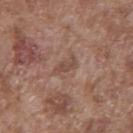Impression: No biopsy was performed on this lesion — it was imaged during a full skin examination and was not determined to be concerning. Background: A male patient in their mid- to late 70s. The lesion's longest dimension is about 3 mm. A roughly 15 mm field-of-view crop from a total-body skin photograph. Imaged with white-light lighting. The lesion is on the chest.